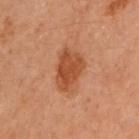This lesion was catalogued during total-body skin photography and was not selected for biopsy. On the left arm. A lesion tile, about 15 mm wide, cut from a 3D total-body photograph. A female patient aged around 60. Imaged with cross-polarized lighting. The total-body-photography lesion software estimated roughly 9 lightness units darker than nearby skin. It also reported border irregularity of about 3 on a 0–10 scale and a peripheral color-asymmetry measure near 1. It also reported a classifier nevus-likeness of about 95/100 and lesion-presence confidence of about 100/100.